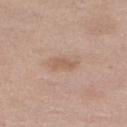biopsy status: catalogued during a skin exam; not biopsied
TBP lesion metrics: a lesion area of about 3 mm² and a shape eccentricity near 0.85; a border-irregularity rating of about 3/10, internal color variation of about 1 on a 0–10 scale, and a peripheral color-asymmetry measure near 0.5
subject: female, aged 53–57
image source: 15 mm crop, total-body photography
size: ~2.5 mm (longest diameter)
body site: the right lower leg
lighting: white-light illumination